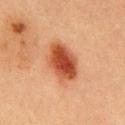Assessment:
No biopsy was performed on this lesion — it was imaged during a full skin examination and was not determined to be concerning.
Background:
Imaged with cross-polarized lighting. On the front of the torso. A male patient about 40 years old. Cropped from a total-body skin-imaging series; the visible field is about 15 mm.